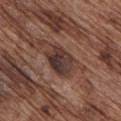Q: Was a biopsy performed?
A: total-body-photography surveillance lesion; no biopsy
Q: What kind of image is this?
A: ~15 mm tile from a whole-body skin photo
Q: How was the tile lit?
A: white-light
Q: What is the lesion's diameter?
A: ~4 mm (longest diameter)
Q: Where on the body is the lesion?
A: the chest
Q: What are the patient's age and sex?
A: male, aged around 75